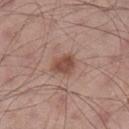<tbp_lesion>
<biopsy_status>not biopsied; imaged during a skin examination</biopsy_status>
<patient>
  <sex>male</sex>
  <age_approx>55</age_approx>
</patient>
<lesion_size>
  <long_diameter_mm_approx>2.5</long_diameter_mm_approx>
</lesion_size>
<lighting>white-light</lighting>
<image>
  <source>total-body photography crop</source>
  <field_of_view_mm>15</field_of_view_mm>
</image>
<automated_metrics>
  <color_variation_0_10>2.5</color_variation_0_10>
  <peripheral_color_asymmetry>1.0</peripheral_color_asymmetry>
  <nevus_likeness_0_100>90</nevus_likeness_0_100>
  <lesion_detection_confidence_0_100>100</lesion_detection_confidence_0_100>
</automated_metrics>
<site>leg</site>
</tbp_lesion>